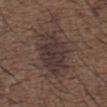Recorded during total-body skin imaging; not selected for excision or biopsy. From the upper back. Approximately 6 mm at its widest. The subject is a male aged approximately 50. A region of skin cropped from a whole-body photographic capture, roughly 15 mm wide. This is a white-light tile. The total-body-photography lesion software estimated an area of roughly 19 mm², a shape eccentricity near 0.5, and a symmetry-axis asymmetry near 0.2. It also reported a border-irregularity index near 3.5/10, a within-lesion color-variation index near 3/10, and radial color variation of about 1. The analysis additionally found an automated nevus-likeness rating near 0 out of 100 and a detector confidence of about 100 out of 100 that the crop contains a lesion.